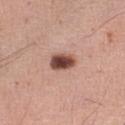<tbp_lesion>
<biopsy_status>not biopsied; imaged during a skin examination</biopsy_status>
<lesion_size>
  <long_diameter_mm_approx>3.5</long_diameter_mm_approx>
</lesion_size>
<site>leg</site>
<automated_metrics>
  <border_irregularity_0_10>1.5</border_irregularity_0_10>
  <color_variation_0_10>5.5</color_variation_0_10>
  <peripheral_color_asymmetry>1.5</peripheral_color_asymmetry>
  <lesion_detection_confidence_0_100>100</lesion_detection_confidence_0_100>
</automated_metrics>
<lighting>white-light</lighting>
<image>
  <source>total-body photography crop</source>
  <field_of_view_mm>15</field_of_view_mm>
</image>
<patient>
  <sex>male</sex>
  <age_approx>60</age_approx>
</patient>
</tbp_lesion>